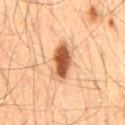Recorded during total-body skin imaging; not selected for excision or biopsy.
The lesion is on the mid back.
About 5 mm across.
Captured under cross-polarized illumination.
A male subject, aged 53–57.
A region of skin cropped from a whole-body photographic capture, roughly 15 mm wide.